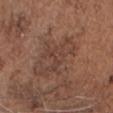Recorded during total-body skin imaging; not selected for excision or biopsy.